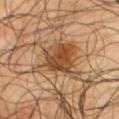Part of a total-body skin-imaging series; this lesion was reviewed on a skin check and was not flagged for biopsy.